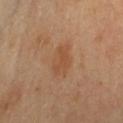Imaged with cross-polarized lighting.
Automated tile analysis of the lesion measured a shape eccentricity near 0.85 and a symmetry-axis asymmetry near 0.25. The analysis additionally found an average lesion color of about L≈51 a*≈21 b*≈34 (CIELAB) and a normalized lesion–skin contrast near 5.5. The software also gave a border-irregularity index near 2.5/10.
A 15 mm close-up extracted from a 3D total-body photography capture.
A female subject aged 38–42.
Measured at roughly 4 mm in maximum diameter.
The lesion is located on the front of the torso.